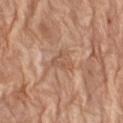| key | value |
|---|---|
| follow-up | catalogued during a skin exam; not biopsied |
| location | the left thigh |
| patient | female, aged around 75 |
| automated metrics | a lesion area of about 4.5 mm², a shape eccentricity near 0.6, and two-axis asymmetry of about 0.55; a lesion color around L≈55 a*≈20 b*≈31 in CIELAB, roughly 7 lightness units darker than nearby skin, and a lesion-to-skin contrast of about 5 (normalized; higher = more distinct); border irregularity of about 5 on a 0–10 scale and a color-variation rating of about 2.5/10; a nevus-likeness score of about 0/100 and a lesion-detection confidence of about 50/100 |
| lesion diameter | ~3 mm (longest diameter) |
| imaging modality | ~15 mm tile from a whole-body skin photo |
| lighting | white-light illumination |